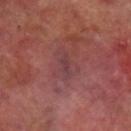Assessment:
Imaged during a routine full-body skin examination; the lesion was not biopsied and no histopathology is available.
Context:
Automated image analysis of the tile measured a shape eccentricity near 0.9 and two-axis asymmetry of about 0.4. And it measured border irregularity of about 4 on a 0–10 scale and a within-lesion color-variation index near 1/10. This is a cross-polarized tile. A roughly 15 mm field-of-view crop from a total-body skin photograph. Longest diameter approximately 3 mm. The lesion is on the right lower leg. A male subject, aged 68–72.